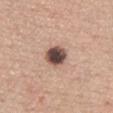This lesion was catalogued during total-body skin photography and was not selected for biopsy.
A male subject, about 45 years old.
The tile uses white-light illumination.
Measured at roughly 3 mm in maximum diameter.
On the left upper arm.
A 15 mm close-up extracted from a 3D total-body photography capture.